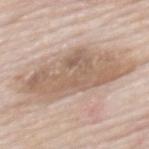Q: Was a biopsy performed?
A: imaged on a skin check; not biopsied
Q: Lesion size?
A: ~10.5 mm (longest diameter)
Q: What is the imaging modality?
A: total-body-photography crop, ~15 mm field of view
Q: What is the anatomic site?
A: the mid back
Q: What are the patient's age and sex?
A: male, approximately 85 years of age or older